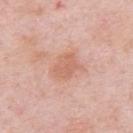Assessment: No biopsy was performed on this lesion — it was imaged during a full skin examination and was not determined to be concerning. Acquisition and patient details: From the right upper arm. A 15 mm close-up tile from a total-body photography series done for melanoma screening. Measured at roughly 3.5 mm in maximum diameter. The lesion-visualizer software estimated a lesion color around L≈63 a*≈24 b*≈30 in CIELAB, a lesion–skin lightness drop of about 8, and a lesion-to-skin contrast of about 5.5 (normalized; higher = more distinct). And it measured a classifier nevus-likeness of about 10/100 and a lesion-detection confidence of about 100/100. The subject is a female aged 63 to 67. Imaged with white-light lighting.